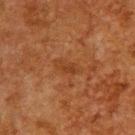Assessment:
Part of a total-body skin-imaging series; this lesion was reviewed on a skin check and was not flagged for biopsy.
Background:
The subject is a male aged approximately 60. Cropped from a total-body skin-imaging series; the visible field is about 15 mm. The recorded lesion diameter is about 3 mm. From the upper back. The tile uses cross-polarized illumination.